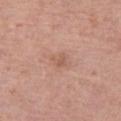biopsy status — no biopsy performed (imaged during a skin exam) | acquisition — 15 mm crop, total-body photography | illumination — white-light illumination | diameter — about 2.5 mm | location — the right thigh | image-analysis metrics — an outline eccentricity of about 0.7 (0 = round, 1 = elongated) and two-axis asymmetry of about 0.3; a lesion color around L≈58 a*≈22 b*≈29 in CIELAB, roughly 7 lightness units darker than nearby skin, and a lesion-to-skin contrast of about 5 (normalized; higher = more distinct) | subject — female, aged 63–67.